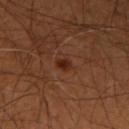Case summary:
* follow-up: catalogued during a skin exam; not biopsied
* automated metrics: a lesion–skin lightness drop of about 8 and a normalized border contrast of about 8.5; a lesion-detection confidence of about 100/100
* patient: male, aged 63–67
* location: the right upper arm
* diameter: ≈2 mm
* illumination: cross-polarized
* imaging modality: total-body-photography crop, ~15 mm field of view